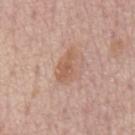The lesion was photographed on a routine skin check and not biopsied; there is no pathology result.
The tile uses white-light illumination.
A male subject in their mid-70s.
From the chest.
A 15 mm crop from a total-body photograph taken for skin-cancer surveillance.
Automated tile analysis of the lesion measured an area of roughly 6.5 mm² and a shape eccentricity near 0.85. The software also gave an average lesion color of about L≈58 a*≈20 b*≈30 (CIELAB), about 8 CIELAB-L* units darker than the surrounding skin, and a lesion-to-skin contrast of about 6.5 (normalized; higher = more distinct). And it measured a border-irregularity index near 4.5/10, a within-lesion color-variation index near 2/10, and a peripheral color-asymmetry measure near 0.5.
Longest diameter approximately 4 mm.